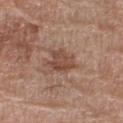* workup — catalogued during a skin exam; not biopsied
* illumination — white-light illumination
* image — ~15 mm tile from a whole-body skin photo
* site — the right thigh
* lesion size — about 3.5 mm
* subject — female, about 80 years old